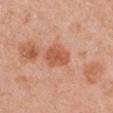Impression:
The lesion was tiled from a total-body skin photograph and was not biopsied.
Clinical summary:
Cropped from a whole-body photographic skin survey; the tile spans about 15 mm. Automated tile analysis of the lesion measured a lesion area of about 7 mm², a shape eccentricity near 0.65, and a symmetry-axis asymmetry near 0.2. It also reported roughly 10 lightness units darker than nearby skin. It also reported a border-irregularity index near 2/10 and radial color variation of about 0.5. About 3.5 mm across. Captured under white-light illumination. A female subject, approximately 50 years of age. On the left upper arm.